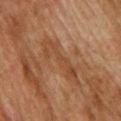The lesion was tiled from a total-body skin photograph and was not biopsied. A roughly 15 mm field-of-view crop from a total-body skin photograph. Imaged with cross-polarized lighting. The lesion's longest dimension is about 6.5 mm. A male subject in their mid-70s. From the upper back.